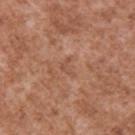| key | value |
|---|---|
| workup | catalogued during a skin exam; not biopsied |